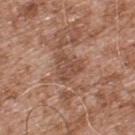Case summary:
• follow-up — imaged on a skin check; not biopsied
• illumination — white-light
• image source — ~15 mm crop, total-body skin-cancer survey
• site — the upper back
• subject — male, in their mid- to late 50s